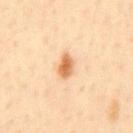Notes:
* workup · total-body-photography surveillance lesion; no biopsy